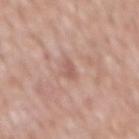biopsy status = no biopsy performed (imaged during a skin exam) | site = the mid back | image = ~15 mm tile from a whole-body skin photo | patient = male, roughly 55 years of age | lesion size = ≈3 mm.